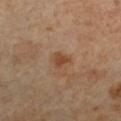This lesion was catalogued during total-body skin photography and was not selected for biopsy.
The tile uses cross-polarized illumination.
The recorded lesion diameter is about 2.5 mm.
A female subject, roughly 50 years of age.
A region of skin cropped from a whole-body photographic capture, roughly 15 mm wide.
Automated tile analysis of the lesion measured an area of roughly 4.5 mm² and two-axis asymmetry of about 0.25. The software also gave a lesion-to-skin contrast of about 7 (normalized; higher = more distinct). And it measured a classifier nevus-likeness of about 75/100.
The lesion is on the left lower leg.